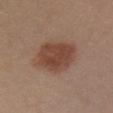workup — total-body-photography surveillance lesion; no biopsy
TBP lesion metrics — two-axis asymmetry of about 0.15; a nevus-likeness score of about 100/100 and a lesion-detection confidence of about 100/100
lesion size — about 6 mm
subject — female, aged approximately 30
imaging modality — total-body-photography crop, ~15 mm field of view
site — the chest